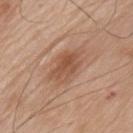Findings:
• notes · no biopsy performed (imaged during a skin exam)
• subject · male, roughly 80 years of age
• body site · the chest
• image · ~15 mm crop, total-body skin-cancer survey
• illumination · white-light
• lesion size · about 5 mm
• image-analysis metrics · a mean CIELAB color near L≈53 a*≈21 b*≈31; a classifier nevus-likeness of about 70/100